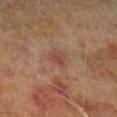Assessment:
This lesion was catalogued during total-body skin photography and was not selected for biopsy.
Context:
About 2.5 mm across. A 15 mm close-up tile from a total-body photography series done for melanoma screening. On the left lower leg. A male patient, approximately 70 years of age. This is a cross-polarized tile.